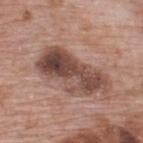Clinical impression:
Imaged during a routine full-body skin examination; the lesion was not biopsied and no histopathology is available.
Image and clinical context:
A male subject, aged approximately 70. Measured at roughly 8 mm in maximum diameter. Located on the upper back. Captured under white-light illumination. A lesion tile, about 15 mm wide, cut from a 3D total-body photograph.